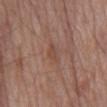Q: Is there a histopathology result?
A: catalogued during a skin exam; not biopsied
Q: Who is the patient?
A: male, approximately 80 years of age
Q: Where on the body is the lesion?
A: the mid back
Q: Illumination type?
A: white-light
Q: What is the imaging modality?
A: 15 mm crop, total-body photography
Q: How large is the lesion?
A: about 3 mm